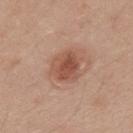Clinical impression: The lesion was photographed on a routine skin check and not biopsied; there is no pathology result. Image and clinical context: Automated image analysis of the tile measured a mean CIELAB color near L≈52 a*≈23 b*≈29, a lesion–skin lightness drop of about 10, and a normalized border contrast of about 7.5. The software also gave a within-lesion color-variation index near 4.5/10 and radial color variation of about 1.5. A 15 mm crop from a total-body photograph taken for skin-cancer surveillance. A male subject aged around 35. This is a white-light tile. Located on the mid back. The recorded lesion diameter is about 4 mm.